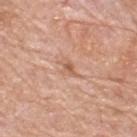Impression: Recorded during total-body skin imaging; not selected for excision or biopsy. Image and clinical context: The lesion's longest dimension is about 2.5 mm. Automated tile analysis of the lesion measured an area of roughly 3 mm² and an outline eccentricity of about 0.75 (0 = round, 1 = elongated). And it measured a lesion color around L≈60 a*≈22 b*≈32 in CIELAB, a lesion–skin lightness drop of about 8, and a lesion-to-skin contrast of about 6 (normalized; higher = more distinct). It also reported a border-irregularity rating of about 3.5/10 and a peripheral color-asymmetry measure near 0.5. The subject is a male roughly 80 years of age. Captured under white-light illumination. A close-up tile cropped from a whole-body skin photograph, about 15 mm across. The lesion is located on the back.